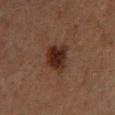Captured during whole-body skin photography for melanoma surveillance; the lesion was not biopsied. The lesion is located on the left lower leg. The subject is a male in their mid-70s. Cropped from a whole-body photographic skin survey; the tile spans about 15 mm.